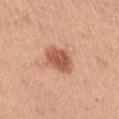Q: Is there a histopathology result?
A: catalogued during a skin exam; not biopsied
Q: How was the tile lit?
A: white-light
Q: What did automated image analysis measure?
A: a lesion color around L≈57 a*≈26 b*≈33 in CIELAB, about 13 CIELAB-L* units darker than the surrounding skin, and a lesion-to-skin contrast of about 8.5 (normalized; higher = more distinct); a border-irregularity index near 2/10, internal color variation of about 3 on a 0–10 scale, and peripheral color asymmetry of about 1; an automated nevus-likeness rating near 95 out of 100 and lesion-presence confidence of about 100/100
Q: What is the lesion's diameter?
A: ≈3.5 mm
Q: Patient demographics?
A: female, in their mid-40s
Q: Where on the body is the lesion?
A: the left upper arm
Q: How was this image acquired?
A: ~15 mm tile from a whole-body skin photo